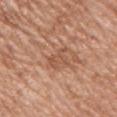{"biopsy_status": "not biopsied; imaged during a skin examination", "lesion_size": {"long_diameter_mm_approx": 2.5}, "patient": {"sex": "male", "age_approx": 55}, "image": {"source": "total-body photography crop", "field_of_view_mm": 15}, "site": "chest", "lighting": "white-light"}